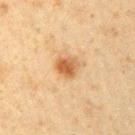<lesion>
<biopsy_status>not biopsied; imaged during a skin examination</biopsy_status>
<patient>
  <sex>male</sex>
  <age_approx>60</age_approx>
</patient>
<site>right upper arm</site>
<lesion_size>
  <long_diameter_mm_approx>3.0</long_diameter_mm_approx>
</lesion_size>
<lighting>cross-polarized</lighting>
<image>
  <source>total-body photography crop</source>
  <field_of_view_mm>15</field_of_view_mm>
</image>
</lesion>